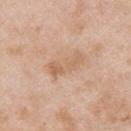{"biopsy_status": "not biopsied; imaged during a skin examination", "lesion_size": {"long_diameter_mm_approx": 4.5}, "image": {"source": "total-body photography crop", "field_of_view_mm": 15}, "lighting": "white-light", "site": "upper back", "patient": {"sex": "male", "age_approx": 25}}